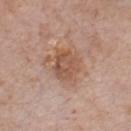Findings:
- workup — catalogued during a skin exam; not biopsied
- image source — total-body-photography crop, ~15 mm field of view
- body site — the chest
- subject — male, aged 73–77
- tile lighting — white-light illumination
- lesion size — about 5 mm